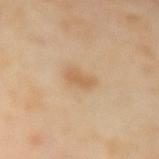| feature | finding |
|---|---|
| workup | total-body-photography surveillance lesion; no biopsy |
| tile lighting | cross-polarized |
| automated metrics | a nevus-likeness score of about 55/100 |
| image source | ~15 mm crop, total-body skin-cancer survey |
| subject | female, about 55 years old |
| location | the arm |
| size | about 3 mm |